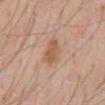Captured during whole-body skin photography for melanoma surveillance; the lesion was not biopsied. Longest diameter approximately 4 mm. An algorithmic analysis of the crop reported an eccentricity of roughly 0.8 and a symmetry-axis asymmetry near 0.25. The analysis additionally found a classifier nevus-likeness of about 45/100 and lesion-presence confidence of about 100/100. The subject is a male aged 58 to 62. From the mid back. Captured under white-light illumination. A close-up tile cropped from a whole-body skin photograph, about 15 mm across.